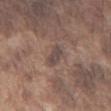Recorded during total-body skin imaging; not selected for excision or biopsy.
From the front of the torso.
The lesion-visualizer software estimated an area of roughly 3.5 mm², a shape eccentricity near 0.9, and two-axis asymmetry of about 0.2. The software also gave border irregularity of about 2.5 on a 0–10 scale, a color-variation rating of about 2.5/10, and a peripheral color-asymmetry measure near 1.
The patient is a male roughly 75 years of age.
The lesion's longest dimension is about 3 mm.
A region of skin cropped from a whole-body photographic capture, roughly 15 mm wide.